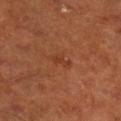The lesion's longest dimension is about 2.5 mm. A male patient aged approximately 70. Located on the right lower leg. A close-up tile cropped from a whole-body skin photograph, about 15 mm across.